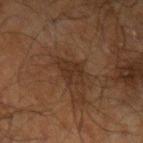This lesion was catalogued during total-body skin photography and was not selected for biopsy.
Imaged with cross-polarized lighting.
About 3 mm across.
Located on the left upper arm.
The lesion-visualizer software estimated a lesion color around L≈24 a*≈14 b*≈23 in CIELAB and roughly 5 lightness units darker than nearby skin. The analysis additionally found a lesion-detection confidence of about 95/100.
A male subject approximately 70 years of age.
This image is a 15 mm lesion crop taken from a total-body photograph.